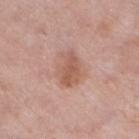Assessment: This lesion was catalogued during total-body skin photography and was not selected for biopsy. Clinical summary: A close-up tile cropped from a whole-body skin photograph, about 15 mm across. Imaged with white-light lighting. A female patient aged approximately 50. From the leg. Automated image analysis of the tile measured an eccentricity of roughly 0.7 and two-axis asymmetry of about 0.25. The software also gave a lesion color around L≈57 a*≈22 b*≈28 in CIELAB and a lesion–skin lightness drop of about 9. The analysis additionally found a border-irregularity rating of about 2.5/10, a within-lesion color-variation index near 3.5/10, and peripheral color asymmetry of about 1.